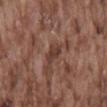follow-up — imaged on a skin check; not biopsied
subject — male, aged 73 to 77
illumination — white-light illumination
size — about 2.5 mm
image source — ~15 mm tile from a whole-body skin photo
automated lesion analysis — a footprint of about 4 mm², a shape eccentricity near 0.6, and a symmetry-axis asymmetry near 0.25; border irregularity of about 2.5 on a 0–10 scale and a within-lesion color-variation index near 2/10
body site — the mid back